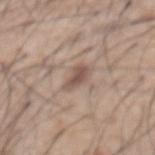follow-up=catalogued during a skin exam; not biopsied
subject=male, roughly 55 years of age
image source=~15 mm crop, total-body skin-cancer survey
body site=the chest